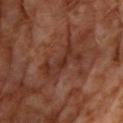Acquisition and patient details:
Automated tile analysis of the lesion measured a within-lesion color-variation index near 3.5/10 and a peripheral color-asymmetry measure near 1. The software also gave a lesion-detection confidence of about 65/100. A 15 mm close-up extracted from a 3D total-body photography capture. A male patient, roughly 60 years of age. The lesion is on the right upper arm. Measured at roughly 5 mm in maximum diameter.